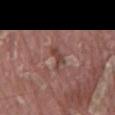Assessment: Captured during whole-body skin photography for melanoma surveillance; the lesion was not biopsied. Clinical summary: The subject is a male aged around 40. Imaged with white-light lighting. Automated image analysis of the tile measured a normalized border contrast of about 7. The analysis additionally found a border-irregularity rating of about 5.5/10, internal color variation of about 0 on a 0–10 scale, and radial color variation of about 0. A close-up tile cropped from a whole-body skin photograph, about 15 mm across. From the mid back.